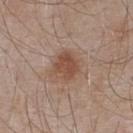Q: Where on the body is the lesion?
A: the upper back
Q: Who is the patient?
A: male, in their mid-50s
Q: How was this image acquired?
A: 15 mm crop, total-body photography